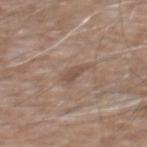biopsy status = imaged on a skin check; not biopsied
patient = male, aged approximately 60
acquisition = ~15 mm tile from a whole-body skin photo
location = the chest